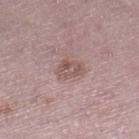{"biopsy_status": "not biopsied; imaged during a skin examination", "site": "right lower leg", "lighting": "white-light", "patient": {"sex": "female", "age_approx": 40}, "lesion_size": {"long_diameter_mm_approx": 3.5}, "image": {"source": "total-body photography crop", "field_of_view_mm": 15}}